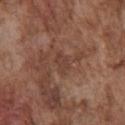Captured during whole-body skin photography for melanoma surveillance; the lesion was not biopsied.
The lesion is located on the chest.
Captured under white-light illumination.
Cropped from a total-body skin-imaging series; the visible field is about 15 mm.
A male patient, roughly 75 years of age.